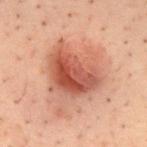No biopsy was performed on this lesion — it was imaged during a full skin examination and was not determined to be concerning.
The total-body-photography lesion software estimated a mean CIELAB color near L≈45 a*≈23 b*≈26, about 11 CIELAB-L* units darker than the surrounding skin, and a lesion-to-skin contrast of about 8.5 (normalized; higher = more distinct). And it measured a border-irregularity index near 5/10, a color-variation rating of about 7.5/10, and radial color variation of about 2.5.
Cropped from a whole-body photographic skin survey; the tile spans about 15 mm.
Located on the mid back.
A male subject, aged approximately 40.
The lesion's longest dimension is about 7.5 mm.